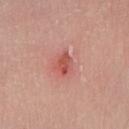The lesion was tiled from a total-body skin photograph and was not biopsied.
The lesion is located on the right lower leg.
A 15 mm crop from a total-body photograph taken for skin-cancer surveillance.
A female subject aged 63–67.
Captured under white-light illumination.
Longest diameter approximately 2.5 mm.
The total-body-photography lesion software estimated about 10 CIELAB-L* units darker than the surrounding skin and a normalized lesion–skin contrast near 7.5. The analysis additionally found a nevus-likeness score of about 0/100 and lesion-presence confidence of about 100/100.